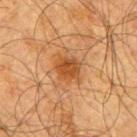A male subject, in their mid-60s.
From the right upper arm.
The recorded lesion diameter is about 3 mm.
A close-up tile cropped from a whole-body skin photograph, about 15 mm across.
This is a cross-polarized tile.